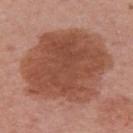Recorded during total-body skin imaging; not selected for excision or biopsy. Cropped from a whole-body photographic skin survey; the tile spans about 15 mm. The lesion is on the left upper arm. Imaged with white-light lighting. A female subject, roughly 55 years of age.